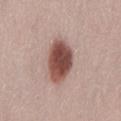biopsy status: no biopsy performed (imaged during a skin exam) | anatomic site: the back | automated metrics: a mean CIELAB color near L≈48 a*≈22 b*≈24, a lesion–skin lightness drop of about 18, and a lesion-to-skin contrast of about 12.5 (normalized; higher = more distinct); a border-irregularity index near 1.5/10 and peripheral color asymmetry of about 1.5; an automated nevus-likeness rating near 100 out of 100 and a lesion-detection confidence of about 100/100 | tile lighting: white-light | size: about 5 mm | acquisition: ~15 mm tile from a whole-body skin photo | subject: female, roughly 50 years of age.